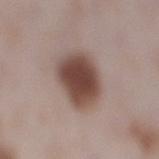Q: Is there a histopathology result?
A: total-body-photography surveillance lesion; no biopsy
Q: What kind of image is this?
A: ~15 mm crop, total-body skin-cancer survey
Q: Who is the patient?
A: female, in their 30s
Q: Lesion location?
A: the leg
Q: What is the lesion's diameter?
A: ≈5.5 mm
Q: How was the tile lit?
A: white-light illumination